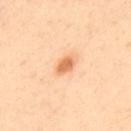Background: Cropped from a total-body skin-imaging series; the visible field is about 15 mm. Automated tile analysis of the lesion measured an average lesion color of about L≈68 a*≈26 b*≈41 (CIELAB), a lesion–skin lightness drop of about 13, and a normalized border contrast of about 8. The software also gave border irregularity of about 2 on a 0–10 scale, internal color variation of about 1.5 on a 0–10 scale, and a peripheral color-asymmetry measure near 0.5. Captured under cross-polarized illumination. The patient is a male in their mid-40s. Located on the upper back. Measured at roughly 2.5 mm in maximum diameter.